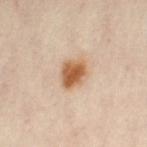No biopsy was performed on this lesion — it was imaged during a full skin examination and was not determined to be concerning.
A female subject aged around 40.
Measured at roughly 4 mm in maximum diameter.
A 15 mm crop from a total-body photograph taken for skin-cancer surveillance.
From the leg.